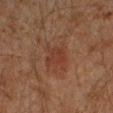biopsy status: no biopsy performed (imaged during a skin exam) | lesion diameter: about 3 mm | automated lesion analysis: a lesion area of about 6 mm² and a shape-asymmetry score of about 0.35 (0 = symmetric); about 5 CIELAB-L* units darker than the surrounding skin and a lesion-to-skin contrast of about 5 (normalized; higher = more distinct); a within-lesion color-variation index near 2/10 and peripheral color asymmetry of about 0.5 | tile lighting: cross-polarized illumination | acquisition: 15 mm crop, total-body photography | location: the left forearm | subject: male, approximately 45 years of age.